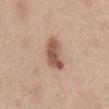Clinical impression: Imaged during a routine full-body skin examination; the lesion was not biopsied and no histopathology is available. Image and clinical context: From the abdomen. A female subject aged 38–42. Automated tile analysis of the lesion measured an area of roughly 9 mm², an eccentricity of roughly 0.85, and two-axis asymmetry of about 0.25. The analysis additionally found a border-irregularity rating of about 2.5/10, a color-variation rating of about 6/10, and a peripheral color-asymmetry measure near 2. The recorded lesion diameter is about 4.5 mm. A 15 mm close-up tile from a total-body photography series done for melanoma screening. The tile uses white-light illumination.